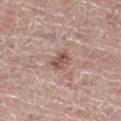Notes:
* notes: imaged on a skin check; not biopsied
* tile lighting: white-light
* patient: female, aged 63–67
* automated metrics: a footprint of about 4.5 mm², an eccentricity of roughly 0.6, and a shape-asymmetry score of about 0.25 (0 = symmetric); an average lesion color of about L≈53 a*≈20 b*≈25 (CIELAB) and a normalized lesion–skin contrast near 7.5; a border-irregularity rating of about 2.5/10, internal color variation of about 4.5 on a 0–10 scale, and a peripheral color-asymmetry measure near 1.5; a lesion-detection confidence of about 100/100
* imaging modality: 15 mm crop, total-body photography
* lesion diameter: ≈3 mm
* anatomic site: the right thigh